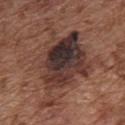{"biopsy_status": "not biopsied; imaged during a skin examination", "lighting": "white-light", "automated_metrics": {"area_mm2_approx": 45.0, "eccentricity": 0.65, "shape_asymmetry": 0.4, "cielab_L": 36, "cielab_a": 18, "cielab_b": 21, "vs_skin_darker_L": 11.0, "border_irregularity_0_10": 8.0, "color_variation_0_10": 10.0, "peripheral_color_asymmetry": 3.5}, "site": "chest", "patient": {"sex": "male", "age_approx": 75}, "image": {"source": "total-body photography crop", "field_of_view_mm": 15}}